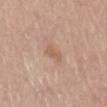The tile uses white-light illumination. The lesion is located on the mid back. Cropped from a total-body skin-imaging series; the visible field is about 15 mm. The subject is a female about 70 years old.